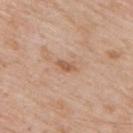Acquisition and patient details: A roughly 15 mm field-of-view crop from a total-body skin photograph. Automated tile analysis of the lesion measured a classifier nevus-likeness of about 5/100 and a lesion-detection confidence of about 100/100. From the upper back. The patient is a male approximately 60 years of age. The recorded lesion diameter is about 2.5 mm. Captured under white-light illumination.